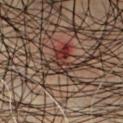No biopsy was performed on this lesion — it was imaged during a full skin examination and was not determined to be concerning. The recorded lesion diameter is about 3.5 mm. On the front of the torso. A lesion tile, about 15 mm wide, cut from a 3D total-body photograph. The subject is a male about 50 years old. The tile uses cross-polarized illumination.